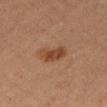follow-up = total-body-photography surveillance lesion; no biopsy
patient = female, aged 38 to 42
imaging modality = ~15 mm crop, total-body skin-cancer survey
body site = the right thigh
lighting = cross-polarized illumination